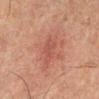{
  "biopsy_status": "not biopsied; imaged during a skin examination",
  "lesion_size": {
    "long_diameter_mm_approx": 3.5
  },
  "lighting": "cross-polarized",
  "image": {
    "source": "total-body photography crop",
    "field_of_view_mm": 15
  },
  "site": "right lower leg",
  "patient": {
    "sex": "male",
    "age_approx": 65
  }
}